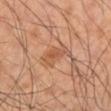biopsy status = total-body-photography surveillance lesion; no biopsy
image = ~15 mm tile from a whole-body skin photo
body site = the right lower leg
patient = male, aged approximately 50
lesion diameter = ≈4 mm
automated lesion analysis = a lesion area of about 5.5 mm², an eccentricity of roughly 0.85, and two-axis asymmetry of about 0.45
illumination = cross-polarized illumination